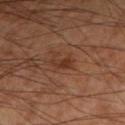workup = imaged on a skin check; not biopsied | acquisition = 15 mm crop, total-body photography | patient = male, aged 58 to 62 | body site = the left lower leg.